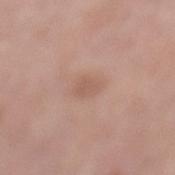Clinical impression:
Part of a total-body skin-imaging series; this lesion was reviewed on a skin check and was not flagged for biopsy.
Clinical summary:
Imaged with white-light lighting. Cropped from a whole-body photographic skin survey; the tile spans about 15 mm. The lesion is located on the left lower leg. Approximately 2.5 mm at its widest. A female patient in their 70s.